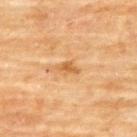Assessment:
No biopsy was performed on this lesion — it was imaged during a full skin examination and was not determined to be concerning.
Context:
The subject is a female about 80 years old. The lesion-visualizer software estimated an eccentricity of roughly 0.8 and two-axis asymmetry of about 0.35. And it measured an average lesion color of about L≈53 a*≈20 b*≈39 (CIELAB) and a lesion-to-skin contrast of about 6.5 (normalized; higher = more distinct). It also reported a color-variation rating of about 0.5/10 and a peripheral color-asymmetry measure near 0. And it measured a classifier nevus-likeness of about 0/100. Captured under cross-polarized illumination. The lesion is on the upper back. Cropped from a whole-body photographic skin survey; the tile spans about 15 mm.